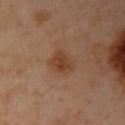Part of a total-body skin-imaging series; this lesion was reviewed on a skin check and was not flagged for biopsy.
A roughly 15 mm field-of-view crop from a total-body skin photograph.
The lesion is located on the left arm.
The lesion's longest dimension is about 3 mm.
An algorithmic analysis of the crop reported an average lesion color of about L≈42 a*≈21 b*≈32 (CIELAB) and a normalized lesion–skin contrast near 7.5. The software also gave internal color variation of about 3 on a 0–10 scale and peripheral color asymmetry of about 1. The software also gave an automated nevus-likeness rating near 60 out of 100.
The subject is a female in their 40s.